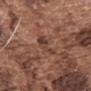Imaged during a routine full-body skin examination; the lesion was not biopsied and no histopathology is available.
A roughly 15 mm field-of-view crop from a total-body skin photograph.
Captured under white-light illumination.
A male patient, aged 73 to 77.
On the upper back.